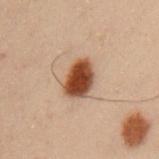  biopsy_status: not biopsied; imaged during a skin examination
  image:
    source: total-body photography crop
    field_of_view_mm: 15
  site: arm
  patient:
    sex: male
    age_approx: 55
  lighting: cross-polarized